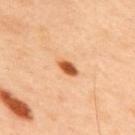Automated tile analysis of the lesion measured a mean CIELAB color near L≈56 a*≈26 b*≈40 and a lesion–skin lightness drop of about 16. And it measured border irregularity of about 2 on a 0–10 scale and internal color variation of about 4 on a 0–10 scale. It also reported a classifier nevus-likeness of about 100/100.
Located on the upper back.
This is a cross-polarized tile.
A male patient, in their mid- to late 40s.
Approximately 3 mm at its widest.
Cropped from a total-body skin-imaging series; the visible field is about 15 mm.